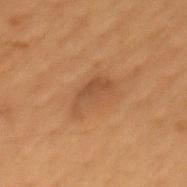{"biopsy_status": "not biopsied; imaged during a skin examination", "lighting": "cross-polarized", "patient": {"sex": "male", "age_approx": 50}, "lesion_size": {"long_diameter_mm_approx": 4.5}, "image": {"source": "total-body photography crop", "field_of_view_mm": 15}, "site": "mid back"}